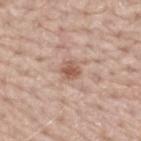Imaged during a routine full-body skin examination; the lesion was not biopsied and no histopathology is available. Captured under white-light illumination. The lesion is located on the mid back. The total-body-photography lesion software estimated an outline eccentricity of about 0.5 (0 = round, 1 = elongated). The software also gave a color-variation rating of about 3/10 and a peripheral color-asymmetry measure near 1. And it measured an automated nevus-likeness rating near 50 out of 100 and a detector confidence of about 100 out of 100 that the crop contains a lesion. This image is a 15 mm lesion crop taken from a total-body photograph. About 2.5 mm across. The subject is a male aged around 65.